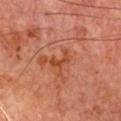Q: Was this lesion biopsied?
A: total-body-photography surveillance lesion; no biopsy
Q: What are the patient's age and sex?
A: male, aged around 65
Q: What lighting was used for the tile?
A: cross-polarized illumination
Q: What kind of image is this?
A: ~15 mm tile from a whole-body skin photo
Q: What is the anatomic site?
A: the chest
Q: Automated lesion metrics?
A: a lesion color around L≈42 a*≈27 b*≈33 in CIELAB, about 7 CIELAB-L* units darker than the surrounding skin, and a lesion-to-skin contrast of about 7 (normalized; higher = more distinct); border irregularity of about 7 on a 0–10 scale, a within-lesion color-variation index near 0/10, and a peripheral color-asymmetry measure near 0; a classifier nevus-likeness of about 0/100 and a lesion-detection confidence of about 100/100
Q: What is the lesion's diameter?
A: ≈3 mm